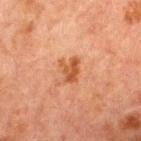Clinical impression:
Imaged during a routine full-body skin examination; the lesion was not biopsied and no histopathology is available.
Background:
A male patient roughly 65 years of age. A roughly 15 mm field-of-view crop from a total-body skin photograph. The lesion is on the chest. The recorded lesion diameter is about 2.5 mm.